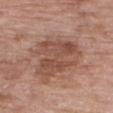automated lesion analysis=a border-irregularity rating of about 7.5/10 and internal color variation of about 4 on a 0–10 scale; a nevus-likeness score of about 10/100 and a detector confidence of about 100 out of 100 that the crop contains a lesion | acquisition=~15 mm crop, total-body skin-cancer survey | lighting=white-light illumination | subject=female, aged around 70 | size=~6 mm (longest diameter) | site=the right upper arm.